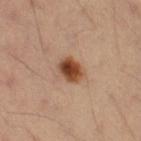Q: Is there a histopathology result?
A: catalogued during a skin exam; not biopsied
Q: What is the anatomic site?
A: the right thigh
Q: Illumination type?
A: cross-polarized
Q: What kind of image is this?
A: ~15 mm tile from a whole-body skin photo
Q: Patient demographics?
A: female, in their mid- to late 30s
Q: What is the lesion's diameter?
A: ~3 mm (longest diameter)